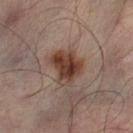Case summary:
* workup · imaged on a skin check; not biopsied
* patient · male, roughly 50 years of age
* lesion size · ≈4 mm
* anatomic site · the left leg
* image · ~15 mm tile from a whole-body skin photo
* TBP lesion metrics · a footprint of about 11 mm², an eccentricity of roughly 0.6, and a symmetry-axis asymmetry near 0.3; border irregularity of about 3 on a 0–10 scale, a color-variation rating of about 6/10, and radial color variation of about 2; a nevus-likeness score of about 95/100
* tile lighting · cross-polarized